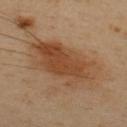Findings:
- notes: total-body-photography surveillance lesion; no biopsy
- patient: male, roughly 50 years of age
- image source: ~15 mm crop, total-body skin-cancer survey
- tile lighting: cross-polarized
- diameter: ≈9 mm
- body site: the upper back